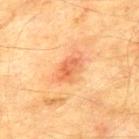| feature | finding |
|---|---|
| workup | total-body-photography surveillance lesion; no biopsy |
| site | the mid back |
| patient | male, aged approximately 75 |
| tile lighting | cross-polarized |
| size | ~3 mm (longest diameter) |
| image source | total-body-photography crop, ~15 mm field of view |
| automated metrics | an area of roughly 5 mm², an outline eccentricity of about 0.8 (0 = round, 1 = elongated), and a shape-asymmetry score of about 0.25 (0 = symmetric); a mean CIELAB color near L≈54 a*≈27 b*≈37, roughly 9 lightness units darker than nearby skin, and a normalized border contrast of about 6; border irregularity of about 2.5 on a 0–10 scale, a within-lesion color-variation index near 5/10, and radial color variation of about 2 |